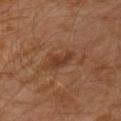Assessment: Part of a total-body skin-imaging series; this lesion was reviewed on a skin check and was not flagged for biopsy. Background: Automated image analysis of the tile measured an area of roughly 4 mm², a shape eccentricity near 0.8, and a shape-asymmetry score of about 0.4 (0 = symmetric). It also reported a border-irregularity rating of about 4/10, internal color variation of about 1.5 on a 0–10 scale, and peripheral color asymmetry of about 0.5. The analysis additionally found an automated nevus-likeness rating near 15 out of 100 and a lesion-detection confidence of about 100/100. Imaged with cross-polarized lighting. Longest diameter approximately 3 mm. The subject is a male aged 28–32. On the left upper arm. A 15 mm crop from a total-body photograph taken for skin-cancer surveillance.